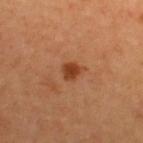image-analysis metrics: an area of roughly 3.5 mm² and two-axis asymmetry of about 0.25; a nevus-likeness score of about 90/100
subject: male, aged approximately 65
body site: the back
lesion diameter: ~2 mm (longest diameter)
tile lighting: cross-polarized
image: total-body-photography crop, ~15 mm field of view